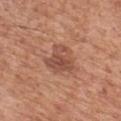On the chest.
The total-body-photography lesion software estimated about 9 CIELAB-L* units darker than the surrounding skin and a normalized lesion–skin contrast near 6.5. The analysis additionally found a border-irregularity index near 4.5/10, internal color variation of about 4.5 on a 0–10 scale, and radial color variation of about 1.5.
A 15 mm close-up tile from a total-body photography series done for melanoma screening.
A male patient, aged 63 to 67.